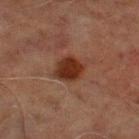Imaged during a routine full-body skin examination; the lesion was not biopsied and no histopathology is available.
The tile uses cross-polarized illumination.
A 15 mm close-up tile from a total-body photography series done for melanoma screening.
The lesion-visualizer software estimated a footprint of about 8.5 mm², an outline eccentricity of about 0.55 (0 = round, 1 = elongated), and two-axis asymmetry of about 0.15. The software also gave an average lesion color of about L≈26 a*≈20 b*≈25 (CIELAB), a lesion–skin lightness drop of about 10, and a lesion-to-skin contrast of about 10.5 (normalized; higher = more distinct). The analysis additionally found a border-irregularity rating of about 1.5/10, a within-lesion color-variation index near 3/10, and peripheral color asymmetry of about 1.
From the left lower leg.
The patient is a male aged 68–72.